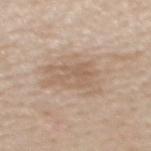Q: Is there a histopathology result?
A: catalogued during a skin exam; not biopsied
Q: What is the anatomic site?
A: the mid back
Q: How large is the lesion?
A: about 4.5 mm
Q: Patient demographics?
A: male, aged approximately 50
Q: What kind of image is this?
A: ~15 mm crop, total-body skin-cancer survey
Q: Illumination type?
A: white-light illumination